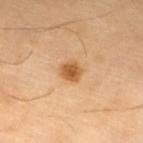workup: total-body-photography surveillance lesion; no biopsy | tile lighting: cross-polarized illumination | site: the left lower leg | acquisition: total-body-photography crop, ~15 mm field of view | patient: female, roughly 70 years of age.